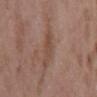Notes:
* follow-up · total-body-photography surveillance lesion; no biopsy
* diameter · ~4.5 mm (longest diameter)
* subject · male, about 50 years old
* image · 15 mm crop, total-body photography
* anatomic site · the mid back
* lighting · white-light